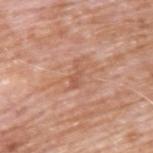A male patient, approximately 60 years of age.
Longest diameter approximately 3 mm.
This image is a 15 mm lesion crop taken from a total-body photograph.
The lesion is on the upper back.
An algorithmic analysis of the crop reported an eccentricity of roughly 0.9 and a shape-asymmetry score of about 0.6 (0 = symmetric). And it measured about 8 CIELAB-L* units darker than the surrounding skin and a lesion-to-skin contrast of about 5.5 (normalized; higher = more distinct). It also reported border irregularity of about 6.5 on a 0–10 scale, a color-variation rating of about 0/10, and peripheral color asymmetry of about 0. The analysis additionally found a nevus-likeness score of about 0/100.
Captured under white-light illumination.